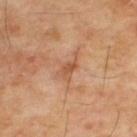{"biopsy_status": "not biopsied; imaged during a skin examination", "image": {"source": "total-body photography crop", "field_of_view_mm": 15}, "site": "upper back", "lesion_size": {"long_diameter_mm_approx": 3.5}, "patient": {"sex": "male", "age_approx": 55}}